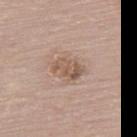Findings:
– workup: imaged on a skin check; not biopsied
– subject: female, approximately 65 years of age
– imaging modality: total-body-photography crop, ~15 mm field of view
– body site: the upper back
– size: ~3.5 mm (longest diameter)